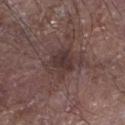Recorded during total-body skin imaging; not selected for excision or biopsy. A male patient, about 80 years old. Located on the left lower leg. Automated image analysis of the tile measured a footprint of about 11 mm² and a shape-asymmetry score of about 0.35 (0 = symmetric). Captured under white-light illumination. Cropped from a whole-body photographic skin survey; the tile spans about 15 mm.